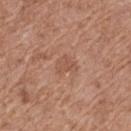biopsy status: imaged on a skin check; not biopsied
location: the left thigh
image-analysis metrics: an area of roughly 4 mm², an outline eccentricity of about 0.65 (0 = round, 1 = elongated), and a shape-asymmetry score of about 0.35 (0 = symmetric); a color-variation rating of about 1.5/10 and radial color variation of about 0.5; a classifier nevus-likeness of about 0/100
lighting: white-light
image source: ~15 mm crop, total-body skin-cancer survey
subject: female, roughly 75 years of age